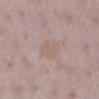Impression:
The lesion was photographed on a routine skin check and not biopsied; there is no pathology result.
Background:
The lesion's longest dimension is about 3.5 mm. Imaged with white-light lighting. The lesion is on the leg. A female patient aged 28–32. This image is a 15 mm lesion crop taken from a total-body photograph. The total-body-photography lesion software estimated a mean CIELAB color near L≈60 a*≈16 b*≈23 and a normalized lesion–skin contrast near 4.5. It also reported an automated nevus-likeness rating near 5 out of 100.